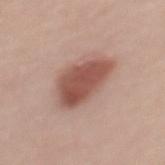<tbp_lesion>
<biopsy_status>not biopsied; imaged during a skin examination</biopsy_status>
<image>
  <source>total-body photography crop</source>
  <field_of_view_mm>15</field_of_view_mm>
</image>
<site>back</site>
<patient>
  <sex>female</sex>
  <age_approx>40</age_approx>
</patient>
</tbp_lesion>